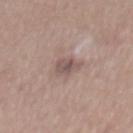follow-up: total-body-photography surveillance lesion; no biopsy | lesion size: ≈3 mm | imaging modality: ~15 mm tile from a whole-body skin photo | site: the mid back | subject: male, in their mid-50s | automated metrics: a mean CIELAB color near L≈52 a*≈17 b*≈20, roughly 9 lightness units darker than nearby skin, and a lesion-to-skin contrast of about 7 (normalized; higher = more distinct); border irregularity of about 2.5 on a 0–10 scale and peripheral color asymmetry of about 1.